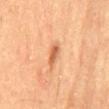• workup — catalogued during a skin exam; not biopsied
• lighting — cross-polarized illumination
• acquisition — ~15 mm crop, total-body skin-cancer survey
• patient — male, aged 58 to 62
• diameter — ~3 mm (longest diameter)